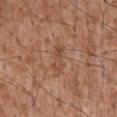  biopsy_status: not biopsied; imaged during a skin examination
  automated_metrics:
    area_mm2_approx: 3.5
    eccentricity: 0.9
    shape_asymmetry: 0.4
  image:
    source: total-body photography crop
    field_of_view_mm: 15
  lesion_size:
    long_diameter_mm_approx: 3.5
  lighting: white-light
  site: chest
  patient:
    sex: male
    age_approx: 45